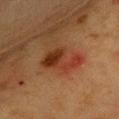Imaged during a routine full-body skin examination; the lesion was not biopsied and no histopathology is available.
Approximately 5 mm at its widest.
From the chest.
A female patient, aged around 55.
Imaged with cross-polarized lighting.
A lesion tile, about 15 mm wide, cut from a 3D total-body photograph.